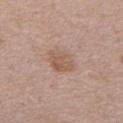Assessment:
Imaged during a routine full-body skin examination; the lesion was not biopsied and no histopathology is available.
Context:
A male subject, about 70 years old. The lesion is on the mid back. A lesion tile, about 15 mm wide, cut from a 3D total-body photograph.